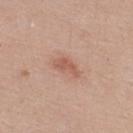Part of a total-body skin-imaging series; this lesion was reviewed on a skin check and was not flagged for biopsy. From the upper back. A male subject aged 38–42. A roughly 15 mm field-of-view crop from a total-body skin photograph.